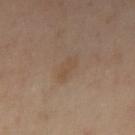Impression: Recorded during total-body skin imaging; not selected for excision or biopsy. Acquisition and patient details: On the left forearm. A female patient aged approximately 40. Automated tile analysis of the lesion measured border irregularity of about 3 on a 0–10 scale. The lesion's longest dimension is about 3 mm. The tile uses cross-polarized illumination. A roughly 15 mm field-of-view crop from a total-body skin photograph.